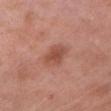Q: Was a biopsy performed?
A: imaged on a skin check; not biopsied
Q: Where on the body is the lesion?
A: the arm
Q: What did automated image analysis measure?
A: a lesion area of about 7 mm², an outline eccentricity of about 0.7 (0 = round, 1 = elongated), and a symmetry-axis asymmetry near 0.2; a color-variation rating of about 2.5/10 and a peripheral color-asymmetry measure near 0.5
Q: What are the patient's age and sex?
A: male, in their mid-50s
Q: What is the lesion's diameter?
A: ~3.5 mm (longest diameter)
Q: Illumination type?
A: white-light illumination
Q: What is the imaging modality?
A: ~15 mm tile from a whole-body skin photo